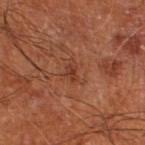The lesion was tiled from a total-body skin photograph and was not biopsied.
A 15 mm crop from a total-body photograph taken for skin-cancer surveillance.
The subject is a male in their mid-60s.
The lesion is on the left lower leg.